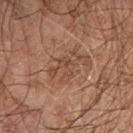This lesion was catalogued during total-body skin photography and was not selected for biopsy. The recorded lesion diameter is about 3 mm. A region of skin cropped from a whole-body photographic capture, roughly 15 mm wide. A male patient, about 70 years old. Located on the right forearm. An algorithmic analysis of the crop reported a lesion–skin lightness drop of about 5 and a normalized border contrast of about 4.5. The analysis additionally found an automated nevus-likeness rating near 0 out of 100. This is a cross-polarized tile.